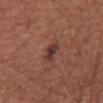Q: What kind of image is this?
A: ~15 mm crop, total-body skin-cancer survey
Q: How was the tile lit?
A: white-light illumination
Q: What are the patient's age and sex?
A: male, roughly 65 years of age
Q: Lesion location?
A: the abdomen